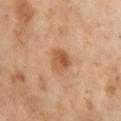Notes:
• notes — total-body-photography surveillance lesion; no biopsy
• image-analysis metrics — an area of roughly 6 mm², an eccentricity of roughly 0.4, and a symmetry-axis asymmetry near 0.25; a nevus-likeness score of about 85/100 and lesion-presence confidence of about 100/100
• anatomic site — the left thigh
• subject — female, roughly 55 years of age
• illumination — cross-polarized illumination
• image — ~15 mm crop, total-body skin-cancer survey